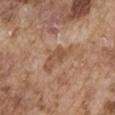From the right upper arm. The recorded lesion diameter is about 4.5 mm. A close-up tile cropped from a whole-body skin photograph, about 15 mm across. A male patient, in their mid- to late 70s.